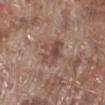Impression:
The lesion was photographed on a routine skin check and not biopsied; there is no pathology result.
Background:
A male patient, aged 68 to 72. Cropped from a total-body skin-imaging series; the visible field is about 15 mm. From the right lower leg.